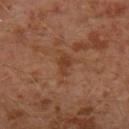Impression:
This lesion was catalogued during total-body skin photography and was not selected for biopsy.
Clinical summary:
A 15 mm crop from a total-body photograph taken for skin-cancer surveillance. A male patient aged 28–32. Located on the left lower leg.